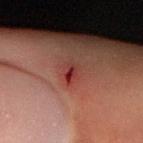Part of a total-body skin-imaging series; this lesion was reviewed on a skin check and was not flagged for biopsy. Cropped from a total-body skin-imaging series; the visible field is about 15 mm. Automated image analysis of the tile measured a border-irregularity index near 2.5/10. It also reported a classifier nevus-likeness of about 0/100. The lesion is on the right forearm. The subject is a male aged around 60. Longest diameter approximately 2.5 mm. Captured under cross-polarized illumination.